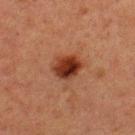notes = catalogued during a skin exam; not biopsied.